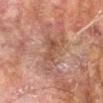Q: Was this lesion biopsied?
A: no biopsy performed (imaged during a skin exam)
Q: What kind of image is this?
A: ~15 mm crop, total-body skin-cancer survey
Q: Where on the body is the lesion?
A: the arm
Q: Who is the patient?
A: male, approximately 75 years of age